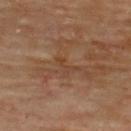<case>
  <biopsy_status>not biopsied; imaged during a skin examination</biopsy_status>
  <automated_metrics>
    <nevus_likeness_0_100>0</nevus_likeness_0_100>
  </automated_metrics>
  <patient>
    <sex>male</sex>
    <age_approx>70</age_approx>
  </patient>
  <image>
    <source>total-body photography crop</source>
    <field_of_view_mm>15</field_of_view_mm>
  </image>
  <site>upper back</site>
  <lesion_size>
    <long_diameter_mm_approx>3.0</long_diameter_mm_approx>
  </lesion_size>
</case>